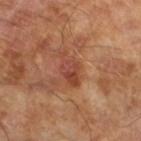Image and clinical context:
A roughly 15 mm field-of-view crop from a total-body skin photograph. A male patient aged approximately 65.